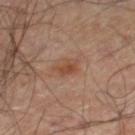Q: Was this lesion biopsied?
A: catalogued during a skin exam; not biopsied
Q: What are the patient's age and sex?
A: male, aged 63 to 67
Q: What did automated image analysis measure?
A: about 8 CIELAB-L* units darker than the surrounding skin and a lesion-to-skin contrast of about 7 (normalized; higher = more distinct); a border-irregularity index near 4/10, a within-lesion color-variation index near 2/10, and radial color variation of about 0.5
Q: What is the lesion's diameter?
A: about 3 mm
Q: What kind of image is this?
A: ~15 mm tile from a whole-body skin photo
Q: Lesion location?
A: the right lower leg